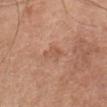workup: imaged on a skin check; not biopsied | tile lighting: white-light | imaging modality: ~15 mm tile from a whole-body skin photo | TBP lesion metrics: an average lesion color of about L≈55 a*≈23 b*≈32 (CIELAB), a lesion–skin lightness drop of about 7, and a normalized lesion–skin contrast near 5 | location: the head or neck | size: about 3 mm | patient: female, roughly 70 years of age.